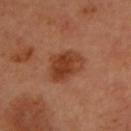The lesion was tiled from a total-body skin photograph and was not biopsied. From the head or neck. The subject is a female aged 58 to 62. Cropped from a total-body skin-imaging series; the visible field is about 15 mm. Longest diameter approximately 5 mm.